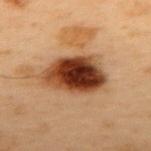Case summary:
• follow-up — no biopsy performed (imaged during a skin exam)
• illumination — cross-polarized
• automated lesion analysis — a mean CIELAB color near L≈32 a*≈21 b*≈29, about 19 CIELAB-L* units darker than the surrounding skin, and a lesion-to-skin contrast of about 15.5 (normalized; higher = more distinct); a border-irregularity index near 2/10, internal color variation of about 9 on a 0–10 scale, and a peripheral color-asymmetry measure near 3; a classifier nevus-likeness of about 100/100
• location — the upper back
• diameter — ≈6.5 mm
• patient — male, aged approximately 55
• acquisition — 15 mm crop, total-body photography